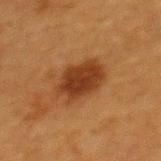Recorded during total-body skin imaging; not selected for excision or biopsy.
Imaged with cross-polarized lighting.
About 5 mm across.
On the upper back.
A female patient aged 38–42.
Automated tile analysis of the lesion measured an area of roughly 13 mm², a shape eccentricity near 0.7, and a shape-asymmetry score of about 0.15 (0 = symmetric). And it measured a color-variation rating of about 2.5/10 and radial color variation of about 1. And it measured a classifier nevus-likeness of about 100/100.
This image is a 15 mm lesion crop taken from a total-body photograph.